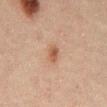Captured during whole-body skin photography for melanoma surveillance; the lesion was not biopsied. Longest diameter approximately 2.5 mm. Imaged with cross-polarized lighting. The lesion is located on the abdomen. A male patient, aged around 50. The total-body-photography lesion software estimated a lesion area of about 3 mm², a shape eccentricity near 0.8, and two-axis asymmetry of about 0.25. And it measured a classifier nevus-likeness of about 70/100 and a lesion-detection confidence of about 100/100. Cropped from a whole-body photographic skin survey; the tile spans about 15 mm.